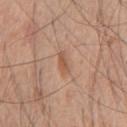notes: total-body-photography surveillance lesion; no biopsy
acquisition: 15 mm crop, total-body photography
lighting: white-light illumination
patient: male, roughly 60 years of age
lesion size: about 2.5 mm
body site: the chest